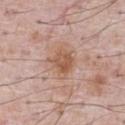Recorded during total-body skin imaging; not selected for excision or biopsy. On the chest. An algorithmic analysis of the crop reported an eccentricity of roughly 0.5 and a shape-asymmetry score of about 0.3 (0 = symmetric). The analysis additionally found border irregularity of about 3.5 on a 0–10 scale and radial color variation of about 1. A male subject aged 68 to 72. A 15 mm close-up tile from a total-body photography series done for melanoma screening.